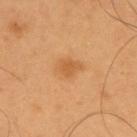Recorded during total-body skin imaging; not selected for excision or biopsy.
Longest diameter approximately 3.5 mm.
Cropped from a total-body skin-imaging series; the visible field is about 15 mm.
Captured under cross-polarized illumination.
A male patient in their mid- to late 50s.
Located on the upper back.